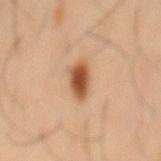Clinical impression:
Recorded during total-body skin imaging; not selected for excision or biopsy.
Image and clinical context:
Cropped from a whole-body photographic skin survey; the tile spans about 15 mm. The patient is a male in their 40s. On the back.